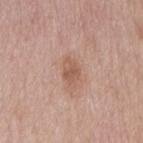Located on the back. A male subject, in their mid- to late 50s. Approximately 3 mm at its widest. A 15 mm crop from a total-body photograph taken for skin-cancer surveillance. The tile uses white-light illumination.